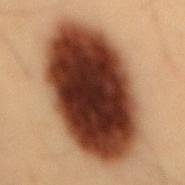biopsy status: catalogued during a skin exam; not biopsied | patient: male, aged approximately 55 | acquisition: 15 mm crop, total-body photography | site: the mid back | size: about 13.5 mm | automated lesion analysis: a footprint of about 75 mm², a shape eccentricity near 0.85, and a shape-asymmetry score of about 0.1 (0 = symmetric); a lesion–skin lightness drop of about 25 and a normalized border contrast of about 20; a border-irregularity index near 1.5/10, internal color variation of about 9 on a 0–10 scale, and radial color variation of about 2.5.